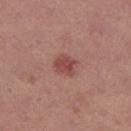Assessment: Imaged during a routine full-body skin examination; the lesion was not biopsied and no histopathology is available. Clinical summary: The total-body-photography lesion software estimated an area of roughly 5.5 mm² and two-axis asymmetry of about 0.2. It also reported an average lesion color of about L≈47 a*≈26 b*≈23 (CIELAB) and a normalized lesion–skin contrast near 7.5. It also reported border irregularity of about 2 on a 0–10 scale, internal color variation of about 2 on a 0–10 scale, and a peripheral color-asymmetry measure near 1. And it measured a nevus-likeness score of about 85/100 and a detector confidence of about 100 out of 100 that the crop contains a lesion. Located on the left thigh. A female subject aged 38 to 42. A region of skin cropped from a whole-body photographic capture, roughly 15 mm wide.